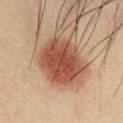Acquisition and patient details: From the chest. Cropped from a total-body skin-imaging series; the visible field is about 15 mm. About 6 mm across. The subject is a male approximately 35 years of age.